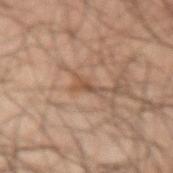{"biopsy_status": "not biopsied; imaged during a skin examination"}